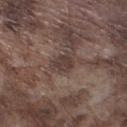The lesion was tiled from a total-body skin photograph and was not biopsied. A male subject, in their mid-70s. From the left lower leg. Automated tile analysis of the lesion measured an area of roughly 4 mm², an outline eccentricity of about 0.7 (0 = round, 1 = elongated), and a symmetry-axis asymmetry near 0.25. The analysis additionally found an automated nevus-likeness rating near 5 out of 100 and lesion-presence confidence of about 85/100. Cropped from a total-body skin-imaging series; the visible field is about 15 mm. Measured at roughly 2.5 mm in maximum diameter. The tile uses white-light illumination.